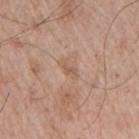| feature | finding |
|---|---|
| notes | imaged on a skin check; not biopsied |
| TBP lesion metrics | a mean CIELAB color near L≈57 a*≈18 b*≈30 and a lesion–skin lightness drop of about 7; a border-irregularity rating of about 3.5/10, a within-lesion color-variation index near 0.5/10, and peripheral color asymmetry of about 0; a classifier nevus-likeness of about 0/100 |
| anatomic site | the right upper arm |
| image source | ~15 mm tile from a whole-body skin photo |
| diameter | about 2.5 mm |
| patient | male, approximately 65 years of age |